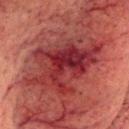Assessment: Imaged during a routine full-body skin examination; the lesion was not biopsied and no histopathology is available.